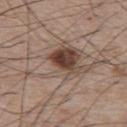follow-up: imaged on a skin check; not biopsied
patient: male, aged 63–67
size: about 10.5 mm
acquisition: 15 mm crop, total-body photography
anatomic site: the upper back